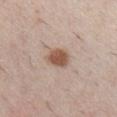  patient:
    sex: male
    age_approx: 25
  site: chest
  image:
    source: total-body photography crop
    field_of_view_mm: 15
  lesion_size:
    long_diameter_mm_approx: 3.0
  lighting: white-light